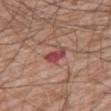<record>
  <biopsy_status>not biopsied; imaged during a skin examination</biopsy_status>
  <lighting>white-light</lighting>
  <patient>
    <sex>male</sex>
    <age_approx>60</age_approx>
  </patient>
  <automated_metrics>
    <cielab_L>45</cielab_L>
    <cielab_a>31</cielab_a>
    <cielab_b>22</cielab_b>
    <vs_skin_contrast_norm>9.5</vs_skin_contrast_norm>
    <color_variation_0_10>2.0</color_variation_0_10>
  </automated_metrics>
  <image>
    <source>total-body photography crop</source>
    <field_of_view_mm>15</field_of_view_mm>
  </image>
  <lesion_size>
    <long_diameter_mm_approx>3.0</long_diameter_mm_approx>
  </lesion_size>
  <site>right thigh</site>
</record>